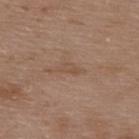{
  "biopsy_status": "not biopsied; imaged during a skin examination",
  "automated_metrics": {
    "color_variation_0_10": 0.5,
    "peripheral_color_asymmetry": 0.5,
    "nevus_likeness_0_100": 0,
    "lesion_detection_confidence_0_100": 100
  },
  "lesion_size": {
    "long_diameter_mm_approx": 3.0
  },
  "lighting": "white-light",
  "site": "upper back",
  "image": {
    "source": "total-body photography crop",
    "field_of_view_mm": 15
  },
  "patient": {
    "sex": "male",
    "age_approx": 50
  }
}